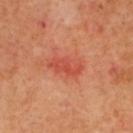| feature | finding |
|---|---|
| workup | total-body-photography surveillance lesion; no biopsy |
| body site | the upper back |
| acquisition | total-body-photography crop, ~15 mm field of view |
| patient | female, roughly 40 years of age |